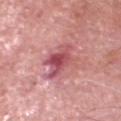This lesion was catalogued during total-body skin photography and was not selected for biopsy.
A roughly 15 mm field-of-view crop from a total-body skin photograph.
The lesion is located on the head or neck.
Longest diameter approximately 4 mm.
The subject is a male aged 68–72.
The tile uses white-light illumination.